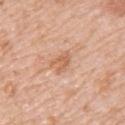workup: no biopsy performed (imaged during a skin exam) | size: ~3 mm (longest diameter) | patient: female, roughly 45 years of age | automated lesion analysis: a footprint of about 4.5 mm², an outline eccentricity of about 0.75 (0 = round, 1 = elongated), and a shape-asymmetry score of about 0.4 (0 = symmetric) | body site: the back | tile lighting: white-light illumination | image source: total-body-photography crop, ~15 mm field of view.